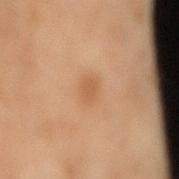Assessment:
Captured during whole-body skin photography for melanoma surveillance; the lesion was not biopsied.
Clinical summary:
Measured at roughly 2.5 mm in maximum diameter. The subject is a male approximately 70 years of age. A roughly 15 mm field-of-view crop from a total-body skin photograph. On the lower back. The tile uses cross-polarized illumination.